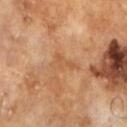The lesion was tiled from a total-body skin photograph and was not biopsied. Automated image analysis of the tile measured a footprint of about 4.5 mm², an eccentricity of roughly 0.9, and two-axis asymmetry of about 0.55. It also reported a lesion color around L≈55 a*≈22 b*≈37 in CIELAB and a lesion-to-skin contrast of about 5 (normalized; higher = more distinct). The software also gave a border-irregularity index near 6/10 and a within-lesion color-variation index near 1.5/10. A 15 mm close-up extracted from a 3D total-body photography capture. A male subject approximately 65 years of age. The recorded lesion diameter is about 4 mm. This is a cross-polarized tile.